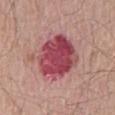Captured during whole-body skin photography for melanoma surveillance; the lesion was not biopsied. Longest diameter approximately 6 mm. Automated tile analysis of the lesion measured a mean CIELAB color near L≈47 a*≈34 b*≈19, about 16 CIELAB-L* units darker than the surrounding skin, and a lesion-to-skin contrast of about 11 (normalized; higher = more distinct). And it measured an automated nevus-likeness rating near 0 out of 100 and lesion-presence confidence of about 100/100. This is a white-light tile. The lesion is located on the back. A male subject about 65 years old. Cropped from a total-body skin-imaging series; the visible field is about 15 mm.